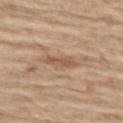Imaged during a routine full-body skin examination; the lesion was not biopsied and no histopathology is available.
A region of skin cropped from a whole-body photographic capture, roughly 15 mm wide.
From the left thigh.
A male patient aged 68 to 72.